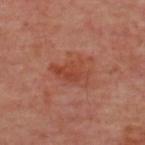workup = catalogued during a skin exam; not biopsied | image source = total-body-photography crop, ~15 mm field of view | subject = approximately 55 years of age | body site = the upper back.